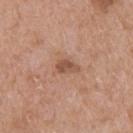<record>
  <biopsy_status>not biopsied; imaged during a skin examination</biopsy_status>
  <automated_metrics>
    <area_mm2_approx>3.5</area_mm2_approx>
    <eccentricity>0.8</eccentricity>
    <border_irregularity_0_10>2.5</border_irregularity_0_10>
    <color_variation_0_10>2.5</color_variation_0_10>
    <peripheral_color_asymmetry>1.0</peripheral_color_asymmetry>
    <nevus_likeness_0_100>15</nevus_likeness_0_100>
    <lesion_detection_confidence_0_100>100</lesion_detection_confidence_0_100>
  </automated_metrics>
  <lighting>white-light</lighting>
  <site>upper back</site>
  <image>
    <source>total-body photography crop</source>
    <field_of_view_mm>15</field_of_view_mm>
  </image>
  <lesion_size>
    <long_diameter_mm_approx>2.5</long_diameter_mm_approx>
  </lesion_size>
  <patient>
    <sex>male</sex>
    <age_approx>65</age_approx>
  </patient>
</record>